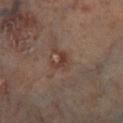<record>
<biopsy_status>not biopsied; imaged during a skin examination</biopsy_status>
<lesion_size>
  <long_diameter_mm_approx>2.5</long_diameter_mm_approx>
</lesion_size>
<site>right lower leg</site>
<image>
  <source>total-body photography crop</source>
  <field_of_view_mm>15</field_of_view_mm>
</image>
<automated_metrics>
  <cielab_L>36</cielab_L>
  <cielab_a>18</cielab_a>
  <cielab_b>23</cielab_b>
  <vs_skin_darker_L>7.0</vs_skin_darker_L>
  <color_variation_0_10>1.5</color_variation_0_10>
  <peripheral_color_asymmetry>0.5</peripheral_color_asymmetry>
  <nevus_likeness_0_100>0</nevus_likeness_0_100>
</automated_metrics>
<patient>
  <sex>male</sex>
  <age_approx>65</age_approx>
</patient>
</record>